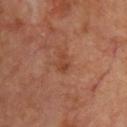Recorded during total-body skin imaging; not selected for excision or biopsy. The subject is a male aged approximately 70. From the upper back. The recorded lesion diameter is about 2.5 mm. A 15 mm crop from a total-body photograph taken for skin-cancer surveillance.